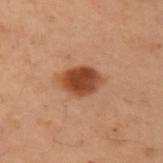Assessment:
The lesion was photographed on a routine skin check and not biopsied; there is no pathology result.
Image and clinical context:
A female subject about 55 years old. The tile uses cross-polarized illumination. A close-up tile cropped from a whole-body skin photograph, about 15 mm across. The lesion is located on the left arm.